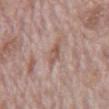<case>
<biopsy_status>not biopsied; imaged during a skin examination</biopsy_status>
<site>abdomen</site>
<image>
  <source>total-body photography crop</source>
  <field_of_view_mm>15</field_of_view_mm>
</image>
<patient>
  <sex>male</sex>
  <age_approx>70</age_approx>
</patient>
<lighting>white-light</lighting>
</case>